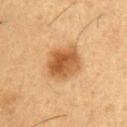The lesion was tiled from a total-body skin photograph and was not biopsied. Automated tile analysis of the lesion measured an area of roughly 14 mm², a shape eccentricity near 0.65, and two-axis asymmetry of about 0.2. The analysis additionally found a lesion color around L≈49 a*≈20 b*≈37 in CIELAB, a lesion–skin lightness drop of about 12, and a lesion-to-skin contrast of about 9 (normalized; higher = more distinct). The software also gave border irregularity of about 2 on a 0–10 scale, a color-variation rating of about 5.5/10, and a peripheral color-asymmetry measure near 2. The analysis additionally found a nevus-likeness score of about 100/100 and a detector confidence of about 100 out of 100 that the crop contains a lesion. A roughly 15 mm field-of-view crop from a total-body skin photograph. The lesion is located on the left upper arm. A male patient approximately 55 years of age. Captured under cross-polarized illumination.